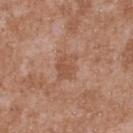biopsy status = imaged on a skin check; not biopsied
lesion size = ≈4 mm
site = the upper back
subject = male, in their mid-40s
tile lighting = white-light
image = ~15 mm tile from a whole-body skin photo